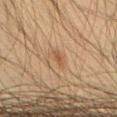No biopsy was performed on this lesion — it was imaged during a full skin examination and was not determined to be concerning. A male subject, aged 33 to 37. Located on the leg. A 15 mm crop from a total-body photograph taken for skin-cancer surveillance.